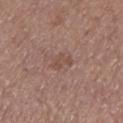Clinical impression:
Recorded during total-body skin imaging; not selected for excision or biopsy.
Acquisition and patient details:
The patient is a female aged approximately 65. A 15 mm crop from a total-body photograph taken for skin-cancer surveillance. Located on the left lower leg.